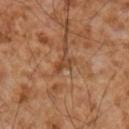Q: Is there a histopathology result?
A: imaged on a skin check; not biopsied
Q: Who is the patient?
A: male, approximately 55 years of age
Q: How was the tile lit?
A: cross-polarized
Q: What kind of image is this?
A: ~15 mm tile from a whole-body skin photo
Q: Where on the body is the lesion?
A: the right upper arm
Q: Lesion size?
A: ~2.5 mm (longest diameter)